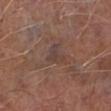<record>
  <biopsy_status>not biopsied; imaged during a skin examination</biopsy_status>
  <image>
    <source>total-body photography crop</source>
    <field_of_view_mm>15</field_of_view_mm>
  </image>
  <patient>
    <sex>male</sex>
    <age_approx>65</age_approx>
  </patient>
  <site>right lower leg</site>
</record>